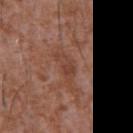  biopsy_status: not biopsied; imaged during a skin examination
  patient:
    sex: male
    age_approx: 45
  lesion_size:
    long_diameter_mm_approx: 4.0
  image:
    source: total-body photography crop
    field_of_view_mm: 15
  lighting: white-light
  site: chest
  automated_metrics:
    area_mm2_approx: 5.5